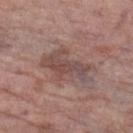Recorded during total-body skin imaging; not selected for excision or biopsy.
Cropped from a whole-body photographic skin survey; the tile spans about 15 mm.
The subject is a female in their mid-80s or older.
The lesion is located on the leg.